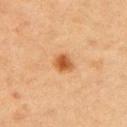Captured during whole-body skin photography for melanoma surveillance; the lesion was not biopsied.
About 2 mm across.
The lesion is located on the left upper arm.
An algorithmic analysis of the crop reported a lesion area of about 4 mm², an eccentricity of roughly 0.35, and a symmetry-axis asymmetry near 0.25. The analysis additionally found a mean CIELAB color near L≈47 a*≈24 b*≈38, roughly 12 lightness units darker than nearby skin, and a normalized border contrast of about 9.5.
A female patient, aged approximately 50.
The tile uses cross-polarized illumination.
A 15 mm close-up tile from a total-body photography series done for melanoma screening.